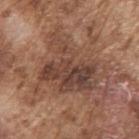The lesion was photographed on a routine skin check and not biopsied; there is no pathology result. Automated tile analysis of the lesion measured about 10 CIELAB-L* units darker than the surrounding skin. And it measured border irregularity of about 8 on a 0–10 scale and peripheral color asymmetry of about 2.5. About 7.5 mm across. A male subject aged 73 to 77. This image is a 15 mm lesion crop taken from a total-body photograph. Located on the right upper arm.